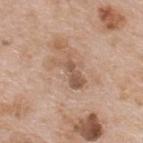Q: Is there a histopathology result?
A: imaged on a skin check; not biopsied
Q: Who is the patient?
A: male, aged around 65
Q: How large is the lesion?
A: ~4.5 mm (longest diameter)
Q: What is the imaging modality?
A: ~15 mm tile from a whole-body skin photo
Q: Lesion location?
A: the upper back
Q: What lighting was used for the tile?
A: white-light